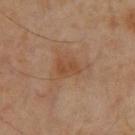biopsy status=total-body-photography surveillance lesion; no biopsy